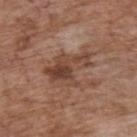workup — imaged on a skin check; not biopsied
patient — male, roughly 70 years of age
lesion size — ≈6 mm
image source — 15 mm crop, total-body photography
image-analysis metrics — a normalized lesion–skin contrast near 7.5; a border-irregularity index near 6/10 and peripheral color asymmetry of about 3; lesion-presence confidence of about 100/100
anatomic site — the upper back